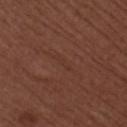Impression: Captured during whole-body skin photography for melanoma surveillance; the lesion was not biopsied. Clinical summary: The subject is a female aged around 50. The tile uses white-light illumination. About 2.5 mm across. From the right upper arm. A 15 mm close-up tile from a total-body photography series done for melanoma screening.